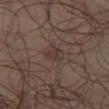biopsy status: no biopsy performed (imaged during a skin exam) | patient: male, approximately 65 years of age | illumination: cross-polarized illumination | lesion size: ~3 mm (longest diameter) | site: the leg | image: 15 mm crop, total-body photography.